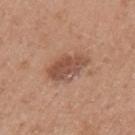{
  "biopsy_status": "not biopsied; imaged during a skin examination",
  "site": "left upper arm",
  "lighting": "white-light",
  "patient": {
    "sex": "female",
    "age_approx": 40
  },
  "image": {
    "source": "total-body photography crop",
    "field_of_view_mm": 15
  },
  "lesion_size": {
    "long_diameter_mm_approx": 4.5
  },
  "automated_metrics": {
    "cielab_L": 51,
    "cielab_a": 21,
    "cielab_b": 29,
    "vs_skin_darker_L": 11.0,
    "vs_skin_contrast_norm": 7.5,
    "border_irregularity_0_10": 2.5,
    "color_variation_0_10": 3.5,
    "nevus_likeness_0_100": 55,
    "lesion_detection_confidence_0_100": 100
  }
}